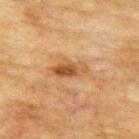Part of a total-body skin-imaging series; this lesion was reviewed on a skin check and was not flagged for biopsy.
A 15 mm close-up extracted from a 3D total-body photography capture.
Located on the upper back.
Captured under cross-polarized illumination.
The patient is a female aged approximately 80.